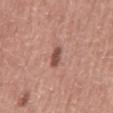| key | value |
|---|---|
| biopsy status | catalogued during a skin exam; not biopsied |
| image-analysis metrics | a border-irregularity index near 2/10, a within-lesion color-variation index near 1/10, and a peripheral color-asymmetry measure near 0.5 |
| location | the mid back |
| lighting | white-light |
| subject | male, aged around 60 |
| lesion diameter | ≈2.5 mm |
| image | total-body-photography crop, ~15 mm field of view |